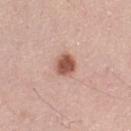biopsy status: no biopsy performed (imaged during a skin exam)
illumination: white-light illumination
diameter: ≈2.5 mm
body site: the left thigh
patient: female, aged 38 to 42
imaging modality: total-body-photography crop, ~15 mm field of view
TBP lesion metrics: a lesion color around L≈54 a*≈24 b*≈28 in CIELAB, a lesion–skin lightness drop of about 16, and a lesion-to-skin contrast of about 10.5 (normalized; higher = more distinct); a color-variation rating of about 3/10 and peripheral color asymmetry of about 1